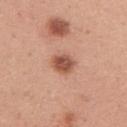• biopsy status — total-body-photography surveillance lesion; no biopsy
• image source — total-body-photography crop, ~15 mm field of view
• patient — female, about 30 years old
• tile lighting — white-light
• automated lesion analysis — an area of roughly 6 mm² and two-axis asymmetry of about 0.2; a border-irregularity index near 2/10 and peripheral color asymmetry of about 1; a lesion-detection confidence of about 100/100
• size — about 3 mm
• site — the arm